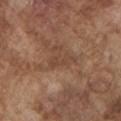<tbp_lesion>
<biopsy_status>not biopsied; imaged during a skin examination</biopsy_status>
<patient>
  <sex>male</sex>
  <age_approx>75</age_approx>
</patient>
<automated_metrics>
  <area_mm2_approx>3.5</area_mm2_approx>
  <eccentricity>0.9</eccentricity>
  <cielab_L>44</cielab_L>
  <cielab_a>19</cielab_a>
  <cielab_b>29</cielab_b>
  <vs_skin_darker_L>6.0</vs_skin_darker_L>
  <border_irregularity_0_10>6.5</border_irregularity_0_10>
  <color_variation_0_10>0.5</color_variation_0_10>
  <peripheral_color_asymmetry>0.0</peripheral_color_asymmetry>
  <nevus_likeness_0_100>0</nevus_likeness_0_100>
</automated_metrics>
<site>left upper arm</site>
<image>
  <source>total-body photography crop</source>
  <field_of_view_mm>15</field_of_view_mm>
</image>
<lighting>white-light</lighting>
<lesion_size>
  <long_diameter_mm_approx>3.5</long_diameter_mm_approx>
</lesion_size>
</tbp_lesion>